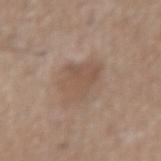Impression: No biopsy was performed on this lesion — it was imaged during a full skin examination and was not determined to be concerning. Background: A male subject, approximately 50 years of age. A lesion tile, about 15 mm wide, cut from a 3D total-body photograph. Approximately 4.5 mm at its widest. On the left forearm.